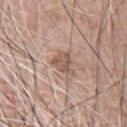workup — imaged on a skin check; not biopsied
subject — male, aged approximately 60
imaging modality — ~15 mm crop, total-body skin-cancer survey
anatomic site — the chest